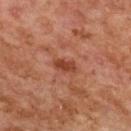The lesion was tiled from a total-body skin photograph and was not biopsied. Measured at roughly 2.5 mm in maximum diameter. The lesion is located on the upper back. A female subject aged approximately 60. A roughly 15 mm field-of-view crop from a total-body skin photograph. Captured under cross-polarized illumination.